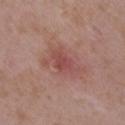Imaged during a routine full-body skin examination; the lesion was not biopsied and no histopathology is available.
A female patient, in their mid-30s.
The lesion is on the right upper arm.
A close-up tile cropped from a whole-body skin photograph, about 15 mm across.